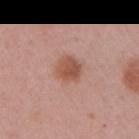Impression: This lesion was catalogued during total-body skin photography and was not selected for biopsy. Acquisition and patient details: A female subject, approximately 55 years of age. A roughly 15 mm field-of-view crop from a total-body skin photograph. The lesion is on the arm. The tile uses white-light illumination.